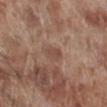Part of a total-body skin-imaging series; this lesion was reviewed on a skin check and was not flagged for biopsy. Approximately 2.5 mm at its widest. On the right lower leg. A 15 mm crop from a total-body photograph taken for skin-cancer surveillance. The subject is a male aged 68–72.